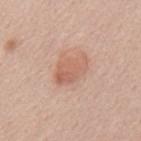{"biopsy_status": "not biopsied; imaged during a skin examination"}